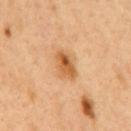Clinical impression: This lesion was catalogued during total-body skin photography and was not selected for biopsy.